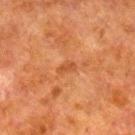The lesion was photographed on a routine skin check and not biopsied; there is no pathology result.
A region of skin cropped from a whole-body photographic capture, roughly 15 mm wide.
Captured under cross-polarized illumination.
Automated tile analysis of the lesion measured about 6 CIELAB-L* units darker than the surrounding skin and a normalized lesion–skin contrast near 5.5. The analysis additionally found border irregularity of about 3 on a 0–10 scale, internal color variation of about 0 on a 0–10 scale, and a peripheral color-asymmetry measure near 0. The analysis additionally found a classifier nevus-likeness of about 0/100 and a detector confidence of about 100 out of 100 that the crop contains a lesion.
The lesion is located on the right lower leg.
A male subject roughly 80 years of age.